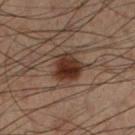This lesion was catalogued during total-body skin photography and was not selected for biopsy.
The lesion is located on the left lower leg.
About 4 mm across.
A region of skin cropped from a whole-body photographic capture, roughly 15 mm wide.
The subject is a male aged 48 to 52.
This is a cross-polarized tile.